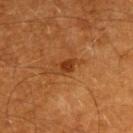Q: Was a biopsy performed?
A: no biopsy performed (imaged during a skin exam)
Q: What kind of image is this?
A: total-body-photography crop, ~15 mm field of view
Q: How large is the lesion?
A: ≈3 mm
Q: What are the patient's age and sex?
A: male, aged 58 to 62
Q: Lesion location?
A: the upper back
Q: Illumination type?
A: cross-polarized illumination